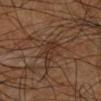Recorded during total-body skin imaging; not selected for excision or biopsy.
On the right lower leg.
A male patient, roughly 60 years of age.
Cropped from a total-body skin-imaging series; the visible field is about 15 mm.